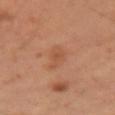Q: Was a biopsy performed?
A: catalogued during a skin exam; not biopsied
Q: What is the anatomic site?
A: the left upper arm
Q: Automated lesion metrics?
A: an outline eccentricity of about 0.95 (0 = round, 1 = elongated) and two-axis asymmetry of about 0.3; an average lesion color of about L≈50 a*≈23 b*≈33 (CIELAB), about 6 CIELAB-L* units darker than the surrounding skin, and a lesion-to-skin contrast of about 5 (normalized; higher = more distinct)
Q: What kind of image is this?
A: ~15 mm crop, total-body skin-cancer survey
Q: Lesion size?
A: ≈4.5 mm
Q: What lighting was used for the tile?
A: cross-polarized illumination
Q: What are the patient's age and sex?
A: male, aged around 55